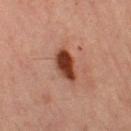Impression: The lesion was tiled from a total-body skin photograph and was not biopsied. Context: A female patient aged approximately 70. Cropped from a whole-body photographic skin survey; the tile spans about 15 mm. The lesion is located on the left thigh. Imaged with cross-polarized lighting.